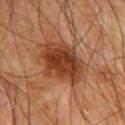* notes · no biopsy performed (imaged during a skin exam)
* subject · male, approximately 65 years of age
* acquisition · total-body-photography crop, ~15 mm field of view
* lighting · cross-polarized
* location · the chest
* TBP lesion metrics · border irregularity of about 3 on a 0–10 scale and a color-variation rating of about 4.5/10